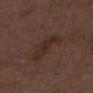This lesion was catalogued during total-body skin photography and was not selected for biopsy. The lesion's longest dimension is about 4.5 mm. Imaged with white-light lighting. A close-up tile cropped from a whole-body skin photograph, about 15 mm across. The subject is a male in their mid-70s. From the head or neck.